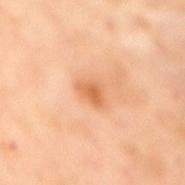Clinical impression:
No biopsy was performed on this lesion — it was imaged during a full skin examination and was not determined to be concerning.
Context:
The lesion is on the mid back. This is a cross-polarized tile. The patient is a female aged 53 to 57. The lesion-visualizer software estimated a nevus-likeness score of about 30/100 and a lesion-detection confidence of about 100/100. A 15 mm close-up extracted from a 3D total-body photography capture.